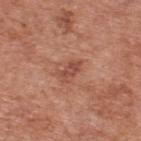| key | value |
|---|---|
| biopsy status | total-body-photography surveillance lesion; no biopsy |
| subject | male, aged approximately 70 |
| location | the upper back |
| diameter | ≈3 mm |
| image-analysis metrics | a lesion area of about 4 mm², a shape eccentricity near 0.85, and two-axis asymmetry of about 0.4; a mean CIELAB color near L≈49 a*≈25 b*≈29, a lesion–skin lightness drop of about 9, and a normalized lesion–skin contrast near 6.5; a nevus-likeness score of about 0/100 and a lesion-detection confidence of about 100/100 |
| imaging modality | ~15 mm crop, total-body skin-cancer survey |
| illumination | white-light illumination |